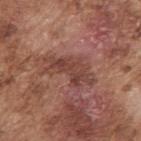biopsy status — total-body-photography surveillance lesion; no biopsy | site — the right upper arm | diameter — ~5.5 mm (longest diameter) | imaging modality — ~15 mm crop, total-body skin-cancer survey | tile lighting — white-light illumination | patient — male, roughly 75 years of age.